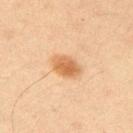Captured during whole-body skin photography for melanoma surveillance; the lesion was not biopsied. This is a cross-polarized tile. A male patient roughly 35 years of age. On the right upper arm. The lesion's longest dimension is about 3.5 mm. A 15 mm close-up extracted from a 3D total-body photography capture. An algorithmic analysis of the crop reported a lesion color around L≈57 a*≈21 b*≈37 in CIELAB and a lesion-to-skin contrast of about 8 (normalized; higher = more distinct). The software also gave a classifier nevus-likeness of about 100/100 and a detector confidence of about 100 out of 100 that the crop contains a lesion.